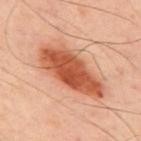Clinical summary: A male patient, aged 48 to 52. Cropped from a total-body skin-imaging series; the visible field is about 15 mm. Automated tile analysis of the lesion measured an area of roughly 25 mm², an eccentricity of roughly 0.9, and a symmetry-axis asymmetry near 0.2. It also reported a mean CIELAB color near L≈55 a*≈29 b*≈36, a lesion–skin lightness drop of about 16, and a normalized lesion–skin contrast near 10.5. And it measured a border-irregularity rating of about 3/10, a color-variation rating of about 5/10, and peripheral color asymmetry of about 1.5. Measured at roughly 8.5 mm in maximum diameter. The lesion is on the upper back. Captured under cross-polarized illumination.